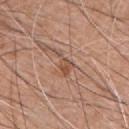– biopsy status — no biopsy performed (imaged during a skin exam)
– site — the chest
– lesion diameter — ≈3.5 mm
– image source — ~15 mm crop, total-body skin-cancer survey
– illumination — white-light illumination
– patient — male, aged approximately 60